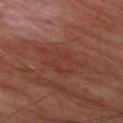<lesion>
  <biopsy_status>not biopsied; imaged during a skin examination</biopsy_status>
  <lesion_size>
    <long_diameter_mm_approx>6.0</long_diameter_mm_approx>
  </lesion_size>
  <patient>
    <sex>male</sex>
    <age_approx>70</age_approx>
  </patient>
  <site>leg</site>
  <image>
    <source>total-body photography crop</source>
    <field_of_view_mm>15</field_of_view_mm>
  </image>
  <automated_metrics>
    <cielab_L>36</cielab_L>
    <cielab_a>24</cielab_a>
    <cielab_b>25</cielab_b>
    <vs_skin_darker_L>5.0</vs_skin_darker_L>
    <vs_skin_contrast_norm>4.5</vs_skin_contrast_norm>
  </automated_metrics>
  <lighting>cross-polarized</lighting>
</lesion>